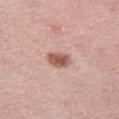Imaged during a routine full-body skin examination; the lesion was not biopsied and no histopathology is available.
A lesion tile, about 15 mm wide, cut from a 3D total-body photograph.
The tile uses white-light illumination.
The recorded lesion diameter is about 3 mm.
A female patient, approximately 65 years of age.
The lesion is on the left thigh.